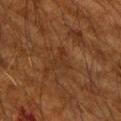notes: no biopsy performed (imaged during a skin exam) | lesion size: ≈3 mm | body site: the right upper arm | patient: male, aged 63–67 | image source: 15 mm crop, total-body photography | illumination: cross-polarized illumination.